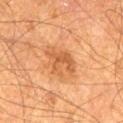workup: catalogued during a skin exam; not biopsied
imaging modality: ~15 mm tile from a whole-body skin photo
location: the left thigh
subject: male, roughly 65 years of age
lighting: cross-polarized illumination
size: ≈4 mm
automated metrics: an area of roughly 7.5 mm² and a symmetry-axis asymmetry near 0.5; a mean CIELAB color near L≈52 a*≈25 b*≈39, roughly 9 lightness units darker than nearby skin, and a lesion-to-skin contrast of about 6.5 (normalized; higher = more distinct)